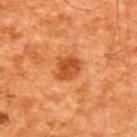* follow-up · total-body-photography surveillance lesion; no biopsy